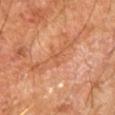Q: What did automated image analysis measure?
A: a shape eccentricity near 0.85 and a shape-asymmetry score of about 0.35 (0 = symmetric); border irregularity of about 4 on a 0–10 scale, internal color variation of about 0 on a 0–10 scale, and peripheral color asymmetry of about 0; a lesion-detection confidence of about 70/100
Q: What are the patient's age and sex?
A: male, about 65 years old
Q: What is the anatomic site?
A: the right upper arm
Q: How was this image acquired?
A: ~15 mm tile from a whole-body skin photo
Q: Lesion size?
A: about 2.5 mm
Q: How was the tile lit?
A: cross-polarized illumination